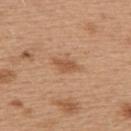Recorded during total-body skin imaging; not selected for excision or biopsy.
The total-body-photography lesion software estimated an eccentricity of roughly 0.85 and a symmetry-axis asymmetry near 0.35. It also reported a border-irregularity rating of about 3.5/10, a within-lesion color-variation index near 0.5/10, and a peripheral color-asymmetry measure near 0.
This is a white-light tile.
From the upper back.
A lesion tile, about 15 mm wide, cut from a 3D total-body photograph.
The recorded lesion diameter is about 3 mm.
A female subject, aged approximately 40.